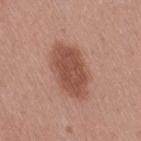Clinical summary:
The total-body-photography lesion software estimated a lesion area of about 20 mm², an eccentricity of roughly 0.8, and two-axis asymmetry of about 0.15. The software also gave a mean CIELAB color near L≈51 a*≈24 b*≈28 and a lesion–skin lightness drop of about 12. The lesion's longest dimension is about 6.5 mm. A close-up tile cropped from a whole-body skin photograph, about 15 mm across. The subject is a female approximately 40 years of age. From the right thigh.